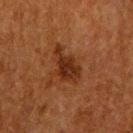No biopsy was performed on this lesion — it was imaged during a full skin examination and was not determined to be concerning. A close-up tile cropped from a whole-body skin photograph, about 15 mm across. A female patient, aged 48 to 52. From the right upper arm.